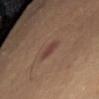The lesion was tiled from a total-body skin photograph and was not biopsied. The lesion is located on the front of the torso. A 15 mm close-up tile from a total-body photography series done for melanoma screening. A female subject roughly 50 years of age. Measured at roughly 2.5 mm in maximum diameter.